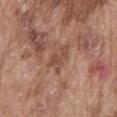biopsy status = no biopsy performed (imaged during a skin exam)